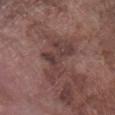Q: Was a biopsy performed?
A: catalogued during a skin exam; not biopsied
Q: What is the lesion's diameter?
A: ~7 mm (longest diameter)
Q: What is the anatomic site?
A: the right lower leg
Q: What did automated image analysis measure?
A: a lesion area of about 14 mm² and a shape eccentricity near 0.9; an average lesion color of about L≈39 a*≈18 b*≈19 (CIELAB), roughly 8 lightness units darker than nearby skin, and a normalized border contrast of about 7; a border-irregularity index near 9/10, internal color variation of about 3.5 on a 0–10 scale, and a peripheral color-asymmetry measure near 1; a nevus-likeness score of about 0/100 and a lesion-detection confidence of about 90/100
Q: Who is the patient?
A: male, aged 73 to 77
Q: How was this image acquired?
A: 15 mm crop, total-body photography
Q: How was the tile lit?
A: white-light